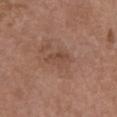The lesion was tiled from a total-body skin photograph and was not biopsied. The subject is a female aged 63–67. Longest diameter approximately 3.5 mm. Imaged with white-light lighting. The total-body-photography lesion software estimated a footprint of about 5.5 mm², an eccentricity of roughly 0.75, and a shape-asymmetry score of about 0.3 (0 = symmetric). And it measured a border-irregularity index near 3/10, internal color variation of about 3 on a 0–10 scale, and a peripheral color-asymmetry measure near 1. The analysis additionally found a detector confidence of about 100 out of 100 that the crop contains a lesion. A close-up tile cropped from a whole-body skin photograph, about 15 mm across. On the chest.